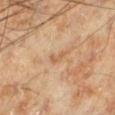Findings:
• follow-up — total-body-photography surveillance lesion; no biopsy
• patient — male, aged 68 to 72
• anatomic site — the left lower leg
• image source — ~15 mm tile from a whole-body skin photo
• lesion diameter — ~2.5 mm (longest diameter)
• lighting — cross-polarized illumination